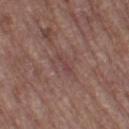No biopsy was performed on this lesion — it was imaged during a full skin examination and was not determined to be concerning.
A 15 mm crop from a total-body photograph taken for skin-cancer surveillance.
Approximately 2.5 mm at its widest.
Automated tile analysis of the lesion measured lesion-presence confidence of about 80/100.
On the left thigh.
Imaged with white-light lighting.
The patient is a female about 65 years old.